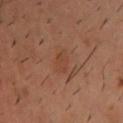follow-up: no biopsy performed (imaged during a skin exam)
acquisition: 15 mm crop, total-body photography
site: the upper back
automated lesion analysis: an outline eccentricity of about 0.8 (0 = round, 1 = elongated) and a symmetry-axis asymmetry near 0.2; roughly 5 lightness units darker than nearby skin and a normalized border contrast of about 4.5; a classifier nevus-likeness of about 0/100 and a lesion-detection confidence of about 100/100
lighting: cross-polarized illumination
patient: male, aged 38 to 42
size: ~3 mm (longest diameter)